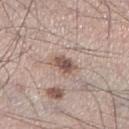Part of a total-body skin-imaging series; this lesion was reviewed on a skin check and was not flagged for biopsy. The lesion is located on the right lower leg. Captured under white-light illumination. A male patient aged around 35. A region of skin cropped from a whole-body photographic capture, roughly 15 mm wide. Approximately 3 mm at its widest. Automated tile analysis of the lesion measured an average lesion color of about L≈53 a*≈17 b*≈23 (CIELAB) and a lesion–skin lightness drop of about 13. The software also gave a nevus-likeness score of about 90/100 and lesion-presence confidence of about 100/100.